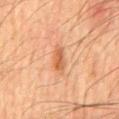<record>
  <biopsy_status>not biopsied; imaged during a skin examination</biopsy_status>
</record>